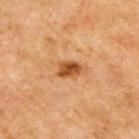follow-up: no biopsy performed (imaged during a skin exam) | subject: male, aged 68–72 | location: the upper back | imaging modality: 15 mm crop, total-body photography.